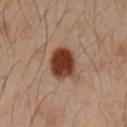- follow-up: total-body-photography surveillance lesion; no biopsy
- patient: male, approximately 50 years of age
- location: the mid back
- diameter: ≈4.5 mm
- lighting: cross-polarized illumination
- automated metrics: an area of roughly 11 mm², a shape eccentricity near 0.7, and a shape-asymmetry score of about 0.1 (0 = symmetric); an average lesion color of about L≈29 a*≈18 b*≈23 (CIELAB), a lesion–skin lightness drop of about 14, and a lesion-to-skin contrast of about 13 (normalized; higher = more distinct); an automated nevus-likeness rating near 100 out of 100
- image source: 15 mm crop, total-body photography